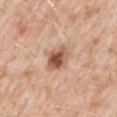{"biopsy_status": "not biopsied; imaged during a skin examination", "image": {"source": "total-body photography crop", "field_of_view_mm": 15}, "automated_metrics": {"area_mm2_approx": 7.5, "eccentricity": 0.45, "shape_asymmetry": 0.2, "color_variation_0_10": 7.0, "peripheral_color_asymmetry": 2.0, "nevus_likeness_0_100": 95}, "lesion_size": {"long_diameter_mm_approx": 3.5}, "patient": {"sex": "male", "age_approx": 50}, "site": "mid back"}